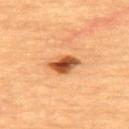| feature | finding |
|---|---|
| acquisition | ~15 mm crop, total-body skin-cancer survey |
| lesion diameter | ≈3.5 mm |
| subject | female, aged approximately 70 |
| illumination | cross-polarized |
| body site | the upper back |